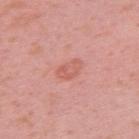biopsy status: catalogued during a skin exam; not biopsied